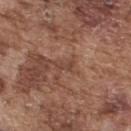The lesion is on the upper back.
The tile uses white-light illumination.
The lesion's longest dimension is about 2.5 mm.
The lesion-visualizer software estimated a lesion–skin lightness drop of about 7 and a normalized border contrast of about 5.5. The analysis additionally found a border-irregularity rating of about 4.5/10, a color-variation rating of about 0/10, and radial color variation of about 0. The software also gave a classifier nevus-likeness of about 0/100 and a lesion-detection confidence of about 85/100.
A male patient, roughly 75 years of age.
A 15 mm crop from a total-body photograph taken for skin-cancer surveillance.